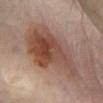workup: catalogued during a skin exam; not biopsied
subject: male, about 70 years old
lesion size: ~11 mm (longest diameter)
tile lighting: cross-polarized
image source: total-body-photography crop, ~15 mm field of view
location: the right lower leg